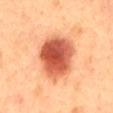Notes:
• biopsy status — imaged on a skin check; not biopsied
• automated metrics — an area of roughly 22 mm², an eccentricity of roughly 0.75, and two-axis asymmetry of about 0.15
• imaging modality — total-body-photography crop, ~15 mm field of view
• illumination — cross-polarized
• body site — the mid back
• subject — male, approximately 50 years of age
• lesion diameter — ≈6.5 mm